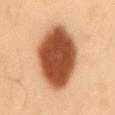Q: Was a biopsy performed?
A: no biopsy performed (imaged during a skin exam)
Q: Illumination type?
A: cross-polarized
Q: What is the imaging modality?
A: ~15 mm crop, total-body skin-cancer survey
Q: What are the patient's age and sex?
A: male, roughly 55 years of age
Q: Lesion size?
A: about 8.5 mm
Q: Lesion location?
A: the mid back